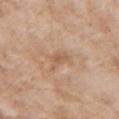Case summary:
– follow-up — imaged on a skin check; not biopsied
– acquisition — 15 mm crop, total-body photography
– TBP lesion metrics — a lesion–skin lightness drop of about 8 and a normalized border contrast of about 5.5; a border-irregularity rating of about 5/10, internal color variation of about 2 on a 0–10 scale, and peripheral color asymmetry of about 0.5; a lesion-detection confidence of about 100/100
– tile lighting — white-light illumination
– lesion diameter — ≈3.5 mm
– patient — female, in their mid-70s
– site — the left upper arm